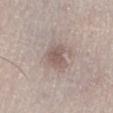- notes · total-body-photography surveillance lesion; no biopsy
- image · 15 mm crop, total-body photography
- body site · the right lower leg
- size · ≈3 mm
- illumination · white-light
- patient · female, approximately 60 years of age